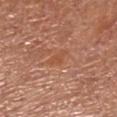Clinical impression:
This lesion was catalogued during total-body skin photography and was not selected for biopsy.
Clinical summary:
Cropped from a whole-body photographic skin survey; the tile spans about 15 mm. The subject is a female aged 63 to 67. On the leg. The lesion's longest dimension is about 3 mm. This is a white-light tile.